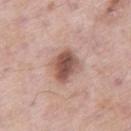Impression:
This lesion was catalogued during total-body skin photography and was not selected for biopsy.
Image and clinical context:
An algorithmic analysis of the crop reported a lesion color around L≈53 a*≈21 b*≈25 in CIELAB, about 15 CIELAB-L* units darker than the surrounding skin, and a normalized lesion–skin contrast near 10. It also reported border irregularity of about 2 on a 0–10 scale, a color-variation rating of about 5/10, and radial color variation of about 1.5. It also reported a nevus-likeness score of about 75/100. A 15 mm close-up tile from a total-body photography series done for melanoma screening. A male patient, aged approximately 55. The recorded lesion diameter is about 3.5 mm. The lesion is located on the leg.